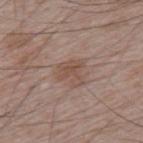The lesion was photographed on a routine skin check and not biopsied; there is no pathology result. Approximately 3 mm at its widest. On the upper back. A male patient aged 63 to 67. A close-up tile cropped from a whole-body skin photograph, about 15 mm across. This is a white-light tile.